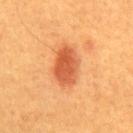Findings:
– notes: catalogued during a skin exam; not biopsied
– patient: male, aged 58–62
– image: 15 mm crop, total-body photography
– body site: the abdomen